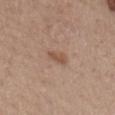Impression:
No biopsy was performed on this lesion — it was imaged during a full skin examination and was not determined to be concerning.
Background:
Imaged with white-light lighting. Measured at roughly 3 mm in maximum diameter. An algorithmic analysis of the crop reported a classifier nevus-likeness of about 10/100. The lesion is located on the back. A 15 mm crop from a total-body photograph taken for skin-cancer surveillance. The patient is a female aged approximately 65.